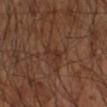Impression:
The lesion was tiled from a total-body skin photograph and was not biopsied.
Clinical summary:
The subject is a male aged around 65. From the left forearm. The tile uses cross-polarized illumination. A lesion tile, about 15 mm wide, cut from a 3D total-body photograph. An algorithmic analysis of the crop reported an area of roughly 3 mm², a shape eccentricity near 0.85, and two-axis asymmetry of about 0.35. The analysis additionally found a lesion color around L≈29 a*≈19 b*≈25 in CIELAB, roughly 5 lightness units darker than nearby skin, and a lesion-to-skin contrast of about 5.5 (normalized; higher = more distinct). And it measured border irregularity of about 4.5 on a 0–10 scale and peripheral color asymmetry of about 0.